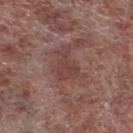– workup — no biopsy performed (imaged during a skin exam)
– patient — male, aged around 55
– tile lighting — white-light illumination
– anatomic site — the leg
– image source — 15 mm crop, total-body photography